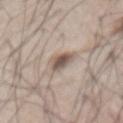The lesion was tiled from a total-body skin photograph and was not biopsied.
A roughly 15 mm field-of-view crop from a total-body skin photograph.
A male subject, aged around 65.
The lesion-visualizer software estimated a footprint of about 5.5 mm², an eccentricity of roughly 0.7, and two-axis asymmetry of about 0.3. It also reported a nevus-likeness score of about 85/100 and a detector confidence of about 85 out of 100 that the crop contains a lesion.
The lesion is located on the front of the torso.
Imaged with white-light lighting.